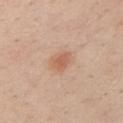{"biopsy_status": "not biopsied; imaged during a skin examination", "automated_metrics": {"area_mm2_approx": 4.5, "shape_asymmetry": 0.25}, "patient": {"sex": "male", "age_approx": 40}, "image": {"source": "total-body photography crop", "field_of_view_mm": 15}, "site": "chest", "lighting": "white-light"}